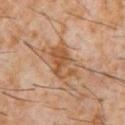The lesion is located on the chest. A male subject, aged 58–62. A roughly 15 mm field-of-view crop from a total-body skin photograph. Imaged with cross-polarized lighting. Automated tile analysis of the lesion measured a lesion–skin lightness drop of about 9 and a normalized lesion–skin contrast near 7.5. It also reported a border-irregularity rating of about 2.5/10 and a within-lesion color-variation index near 5.5/10. The recorded lesion diameter is about 4.5 mm.